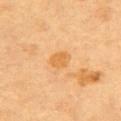{"patient": {"sex": "female", "age_approx": 60}, "image": {"source": "total-body photography crop", "field_of_view_mm": 15}, "site": "back"}